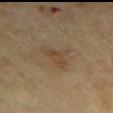Background:
About 4.5 mm across. A close-up tile cropped from a whole-body skin photograph, about 15 mm across. From the right upper arm. A male patient, aged 68 to 72. This is a cross-polarized tile.